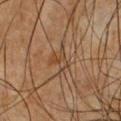notes: total-body-photography surveillance lesion; no biopsy
size: ~3 mm (longest diameter)
illumination: cross-polarized illumination
patient: male, aged 48–52
acquisition: ~15 mm tile from a whole-body skin photo
body site: the chest
TBP lesion metrics: a nevus-likeness score of about 0/100